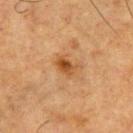| field | value |
|---|---|
| follow-up | total-body-photography surveillance lesion; no biopsy |
| lighting | cross-polarized |
| site | the chest |
| automated lesion analysis | a nevus-likeness score of about 55/100 |
| imaging modality | total-body-photography crop, ~15 mm field of view |
| patient | male, roughly 65 years of age |